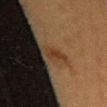Part of a total-body skin-imaging series; this lesion was reviewed on a skin check and was not flagged for biopsy. On the abdomen. The subject is a female aged 28 to 32. A lesion tile, about 15 mm wide, cut from a 3D total-body photograph. This is a cross-polarized tile.